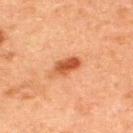A male patient aged 48 to 52. Measured at roughly 3.5 mm in maximum diameter. Automated image analysis of the tile measured roughly 11 lightness units darker than nearby skin and a normalized border contrast of about 9. The software also gave a color-variation rating of about 4.5/10 and peripheral color asymmetry of about 1.5. The tile uses cross-polarized illumination. Located on the upper back. A 15 mm close-up tile from a total-body photography series done for melanoma screening.